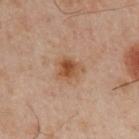This is a cross-polarized tile.
An algorithmic analysis of the crop reported a footprint of about 6.5 mm² and two-axis asymmetry of about 0.25. And it measured a mean CIELAB color near L≈41 a*≈17 b*≈28, roughly 8 lightness units darker than nearby skin, and a normalized lesion–skin contrast near 8. It also reported lesion-presence confidence of about 100/100.
The subject is a male aged 48 to 52.
The lesion is located on the arm.
A close-up tile cropped from a whole-body skin photograph, about 15 mm across.
About 3 mm across.